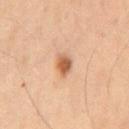No biopsy was performed on this lesion — it was imaged during a full skin examination and was not determined to be concerning. A male subject, approximately 55 years of age. This is a cross-polarized tile. On the abdomen. A 15 mm close-up extracted from a 3D total-body photography capture. Automated tile analysis of the lesion measured a footprint of about 4.5 mm², a shape eccentricity near 0.7, and a symmetry-axis asymmetry near 0.2. Measured at roughly 3 mm in maximum diameter.